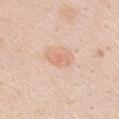<tbp_lesion>
<biopsy_status>not biopsied; imaged during a skin examination</biopsy_status>
<lighting>white-light</lighting>
<automated_metrics>
  <area_mm2_approx>3.5</area_mm2_approx>
  <eccentricity>0.8</eccentricity>
  <nevus_likeness_0_100>55</nevus_likeness_0_100>
  <lesion_detection_confidence_0_100>100</lesion_detection_confidence_0_100>
</automated_metrics>
<site>front of the torso</site>
<image>
  <source>total-body photography crop</source>
  <field_of_view_mm>15</field_of_view_mm>
</image>
<patient>
  <sex>female</sex>
  <age_approx>45</age_approx>
</patient>
</tbp_lesion>